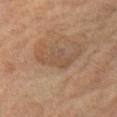workup = catalogued during a skin exam; not biopsied | lesion size = about 5 mm | site = the leg | acquisition = 15 mm crop, total-body photography | lighting = cross-polarized illumination | patient = female, aged around 70.